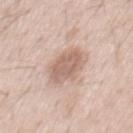Impression: Part of a total-body skin-imaging series; this lesion was reviewed on a skin check and was not flagged for biopsy. Context: The lesion is on the mid back. The tile uses white-light illumination. A lesion tile, about 15 mm wide, cut from a 3D total-body photograph. A male patient, in their 50s. Approximately 5 mm at its widest.